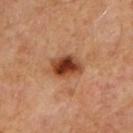Longest diameter approximately 4 mm. A male subject, aged around 65. This is a cross-polarized tile. From the upper back. A region of skin cropped from a whole-body photographic capture, roughly 15 mm wide.